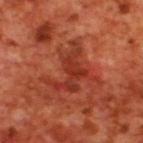This lesion was catalogued during total-body skin photography and was not selected for biopsy.
Automated tile analysis of the lesion measured a lesion area of about 13 mm², an eccentricity of roughly 0.6, and two-axis asymmetry of about 0.7. The analysis additionally found a mean CIELAB color near L≈36 a*≈33 b*≈33, about 9 CIELAB-L* units darker than the surrounding skin, and a normalized lesion–skin contrast near 7. It also reported a border-irregularity index near 10/10, a within-lesion color-variation index near 3/10, and a peripheral color-asymmetry measure near 1.
On the upper back.
A 15 mm close-up extracted from a 3D total-body photography capture.
A male patient, aged 68–72.
Approximately 5.5 mm at its widest.